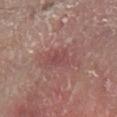Findings:
– notes · imaged on a skin check; not biopsied
– location · the right lower leg
– image source · 15 mm crop, total-body photography
– size · ≈3 mm
– subject · male, aged 78 to 82